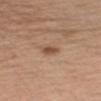– biopsy status — catalogued during a skin exam; not biopsied